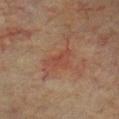Findings:
- workup · no biopsy performed (imaged during a skin exam)
- patient · male, aged around 75
- size · ≈3.5 mm
- tile lighting · cross-polarized illumination
- acquisition · ~15 mm tile from a whole-body skin photo
- site · the chest